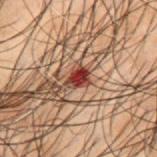notes = total-body-photography surveillance lesion; no biopsy
acquisition = 15 mm crop, total-body photography
location = the upper back
patient = male, aged 48 to 52